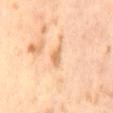{"biopsy_status": "not biopsied; imaged during a skin examination", "image": {"source": "total-body photography crop", "field_of_view_mm": 15}, "patient": {"sex": "male", "age_approx": 65}, "lighting": "cross-polarized", "automated_metrics": {"area_mm2_approx": 3.0, "eccentricity": 0.85, "shape_asymmetry": 0.35, "vs_skin_contrast_norm": 7.0, "nevus_likeness_0_100": 0, "lesion_detection_confidence_0_100": 100}, "lesion_size": {"long_diameter_mm_approx": 2.5}}